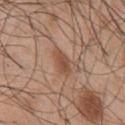Case summary:
- biopsy status · total-body-photography surveillance lesion; no biopsy
- TBP lesion metrics · a lesion color around L≈50 a*≈22 b*≈31 in CIELAB, a lesion–skin lightness drop of about 9, and a normalized border contrast of about 7; border irregularity of about 2 on a 0–10 scale and radial color variation of about 1
- lighting · white-light illumination
- location · the chest
- patient · male, about 45 years old
- imaging modality · ~15 mm tile from a whole-body skin photo